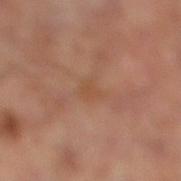| field | value |
|---|---|
| biopsy status | no biopsy performed (imaged during a skin exam) |
| automated lesion analysis | a lesion area of about 3.5 mm², an eccentricity of roughly 0.8, and two-axis asymmetry of about 0.4; a lesion color around L≈47 a*≈20 b*≈31 in CIELAB, a lesion–skin lightness drop of about 5, and a normalized lesion–skin contrast near 4.5; a border-irregularity index near 4.5/10 and peripheral color asymmetry of about 0.5; an automated nevus-likeness rating near 0 out of 100 and lesion-presence confidence of about 100/100 |
| acquisition | ~15 mm tile from a whole-body skin photo |
| illumination | cross-polarized |
| diameter | about 3 mm |
| site | the right lower leg |
| patient | male, in their mid-60s |